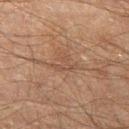<lesion>
  <patient>
    <sex>male</sex>
    <age_approx>60</age_approx>
  </patient>
  <image>
    <source>total-body photography crop</source>
    <field_of_view_mm>15</field_of_view_mm>
  </image>
  <site>left thigh</site>
</lesion>